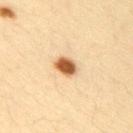{
  "biopsy_status": "not biopsied; imaged during a skin examination",
  "patient": {
    "sex": "female",
    "age_approx": 30
  },
  "site": "right upper arm",
  "image": {
    "source": "total-body photography crop",
    "field_of_view_mm": 15
  }
}